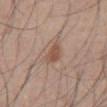Clinical impression: Recorded during total-body skin imaging; not selected for excision or biopsy. Clinical summary: The lesion is located on the abdomen. The subject is a male in their 60s. Imaged with white-light lighting. About 3.5 mm across. Cropped from a total-body skin-imaging series; the visible field is about 15 mm. Automated image analysis of the tile measured a within-lesion color-variation index near 3/10 and a peripheral color-asymmetry measure near 1.